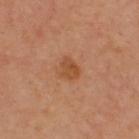Imaged during a routine full-body skin examination; the lesion was not biopsied and no histopathology is available. A roughly 15 mm field-of-view crop from a total-body skin photograph. From the back. The lesion's longest dimension is about 2.5 mm. Imaged with cross-polarized lighting. A female subject aged 43 to 47.